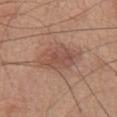Impression: The lesion was photographed on a routine skin check and not biopsied; there is no pathology result. Context: A 15 mm close-up tile from a total-body photography series done for melanoma screening. The lesion is located on the chest. A male patient, aged around 75.